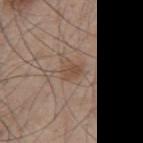– notes — imaged on a skin check; not biopsied
– location — the mid back
– acquisition — 15 mm crop, total-body photography
– patient — male, approximately 55 years of age